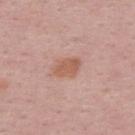Clinical impression: Recorded during total-body skin imaging; not selected for excision or biopsy. Clinical summary: The tile uses white-light illumination. The recorded lesion diameter is about 3.5 mm. The lesion is located on the upper back. A 15 mm close-up tile from a total-body photography series done for melanoma screening. A male patient in their 50s.